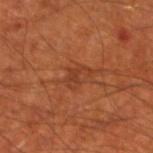workup: catalogued during a skin exam; not biopsied
subject: male, aged 68 to 72
image: total-body-photography crop, ~15 mm field of view
location: the left lower leg
lesion size: ≈3 mm
lighting: cross-polarized illumination